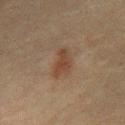Impression: Imaged during a routine full-body skin examination; the lesion was not biopsied and no histopathology is available. Image and clinical context: This is a cross-polarized tile. The recorded lesion diameter is about 4.5 mm. A male subject, aged approximately 60. A 15 mm close-up extracted from a 3D total-body photography capture. The lesion-visualizer software estimated a color-variation rating of about 1.5/10 and a peripheral color-asymmetry measure near 0.5. From the chest.